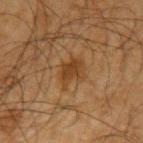Findings:
* notes — imaged on a skin check; not biopsied
* automated lesion analysis — an outline eccentricity of about 0.7 (0 = round, 1 = elongated) and a symmetry-axis asymmetry near 0.35; border irregularity of about 4 on a 0–10 scale and a color-variation rating of about 2/10; an automated nevus-likeness rating near 65 out of 100
* imaging modality — total-body-photography crop, ~15 mm field of view
* lighting — cross-polarized
* patient — male, in their mid- to late 60s
* size — about 3.5 mm
* site — the left upper arm